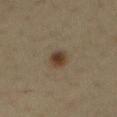Impression:
No biopsy was performed on this lesion — it was imaged during a full skin examination and was not determined to be concerning.
Context:
About 3 mm across. Cropped from a whole-body photographic skin survey; the tile spans about 15 mm. The lesion is located on the abdomen. A male subject, in their mid-30s.